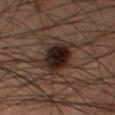{
  "biopsy_status": "not biopsied; imaged during a skin examination",
  "image": {
    "source": "total-body photography crop",
    "field_of_view_mm": 15
  },
  "automated_metrics": {
    "nevus_likeness_0_100": 85,
    "lesion_detection_confidence_0_100": 100
  },
  "patient": {
    "sex": "male",
    "age_approx": 55
  },
  "lighting": "cross-polarized",
  "site": "right thigh",
  "lesion_size": {
    "long_diameter_mm_approx": 7.0
  }
}